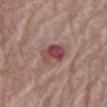{
  "biopsy_status": "not biopsied; imaged during a skin examination",
  "image": {
    "source": "total-body photography crop",
    "field_of_view_mm": 15
  },
  "site": "abdomen",
  "lighting": "white-light",
  "automated_metrics": {
    "nevus_likeness_0_100": 0,
    "lesion_detection_confidence_0_100": 100
  },
  "lesion_size": {
    "long_diameter_mm_approx": 4.0
  },
  "patient": {
    "sex": "female",
    "age_approx": 65
  }
}